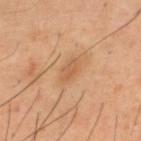Clinical impression: Imaged during a routine full-body skin examination; the lesion was not biopsied and no histopathology is available. Image and clinical context: A male patient, roughly 40 years of age. The tile uses cross-polarized illumination. Automated image analysis of the tile measured an average lesion color of about L≈57 a*≈21 b*≈36 (CIELAB) and a lesion-to-skin contrast of about 5 (normalized; higher = more distinct). It also reported a border-irregularity index near 3.5/10, internal color variation of about 1 on a 0–10 scale, and a peripheral color-asymmetry measure near 0.5. And it measured a nevus-likeness score of about 10/100 and lesion-presence confidence of about 100/100. This image is a 15 mm lesion crop taken from a total-body photograph. About 2.5 mm across. The lesion is on the upper back.